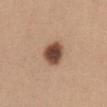The lesion-visualizer software estimated a mean CIELAB color near L≈47 a*≈20 b*≈28, roughly 18 lightness units darker than nearby skin, and a lesion-to-skin contrast of about 12.5 (normalized; higher = more distinct). It also reported a color-variation rating of about 4.5/10 and a peripheral color-asymmetry measure near 1.
This image is a 15 mm lesion crop taken from a total-body photograph.
The lesion is located on the abdomen.
About 3.5 mm across.
The subject is a female in their mid-50s.